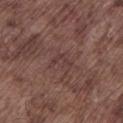Assessment:
The lesion was tiled from a total-body skin photograph and was not biopsied.
Background:
From the left lower leg. The lesion's longest dimension is about 3 mm. This image is a 15 mm lesion crop taken from a total-body photograph. A male subject, aged approximately 75. Automated image analysis of the tile measured an outline eccentricity of about 0.85 (0 = round, 1 = elongated). It also reported a nevus-likeness score of about 0/100 and a detector confidence of about 55 out of 100 that the crop contains a lesion. This is a white-light tile.